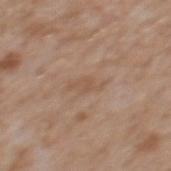Impression: No biopsy was performed on this lesion — it was imaged during a full skin examination and was not determined to be concerning. Background: A male patient aged 63–67. The lesion is on the mid back. This image is a 15 mm lesion crop taken from a total-body photograph.